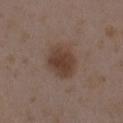Clinical impression: The lesion was tiled from a total-body skin photograph and was not biopsied. Image and clinical context: This image is a 15 mm lesion crop taken from a total-body photograph. Approximately 4.5 mm at its widest. A female subject in their mid- to late 30s. On the left upper arm.